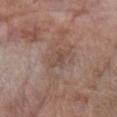{
  "biopsy_status": "not biopsied; imaged during a skin examination",
  "image": {
    "source": "total-body photography crop",
    "field_of_view_mm": 15
  },
  "lesion_size": {
    "long_diameter_mm_approx": 3.0
  },
  "lighting": "white-light",
  "automated_metrics": {
    "eccentricity": 0.8,
    "shape_asymmetry": 0.25,
    "vs_skin_darker_L": 6.0,
    "vs_skin_contrast_norm": 5.0,
    "nevus_likeness_0_100": 0,
    "lesion_detection_confidence_0_100": 100
  },
  "site": "left forearm",
  "patient": {
    "sex": "female",
    "age_approx": 65
  }
}